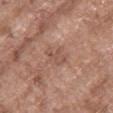Findings:
* biopsy status · imaged on a skin check; not biopsied
* patient · female, roughly 65 years of age
* image · total-body-photography crop, ~15 mm field of view
* lesion size · ~3 mm (longest diameter)
* automated lesion analysis · an average lesion color of about L≈52 a*≈20 b*≈27 (CIELAB) and a normalized lesion–skin contrast near 5; peripheral color asymmetry of about 1
* site · the chest
* tile lighting · white-light illumination